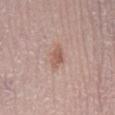Notes:
– notes: total-body-photography surveillance lesion; no biopsy
– site: the right lower leg
– lighting: white-light
– image: ~15 mm tile from a whole-body skin photo
– subject: male, about 80 years old
– lesion diameter: about 2.5 mm